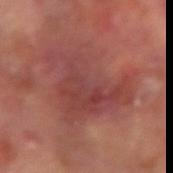Part of a total-body skin-imaging series; this lesion was reviewed on a skin check and was not flagged for biopsy.
The lesion is on the left forearm.
The patient is a male aged 63–67.
Cropped from a whole-body photographic skin survey; the tile spans about 15 mm.
Imaged with cross-polarized lighting.
The lesion-visualizer software estimated a footprint of about 32 mm².
The lesion's longest dimension is about 9 mm.